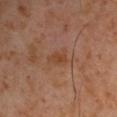workup: no biopsy performed (imaged during a skin exam) | subject: male, roughly 60 years of age | acquisition: 15 mm crop, total-body photography | automated metrics: an area of roughly 4 mm², an outline eccentricity of about 0.75 (0 = round, 1 = elongated), and a symmetry-axis asymmetry near 0.4; a lesion–skin lightness drop of about 6; a border-irregularity index near 4.5/10, a within-lesion color-variation index near 2.5/10, and peripheral color asymmetry of about 1; a nevus-likeness score of about 5/100 and a detector confidence of about 100 out of 100 that the crop contains a lesion | diameter: about 2.5 mm | illumination: cross-polarized illumination | body site: the left upper arm.